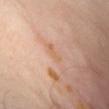follow-up: imaged on a skin check; not biopsied | lesion diameter: about 3 mm | location: the abdomen | illumination: cross-polarized | image: total-body-photography crop, ~15 mm field of view | subject: male, about 65 years old.